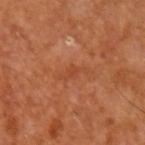workup = total-body-photography surveillance lesion; no biopsy
anatomic site = the left upper arm
lighting = cross-polarized
patient = aged 63 to 67
size = ≈3 mm
imaging modality = 15 mm crop, total-body photography
automated lesion analysis = a color-variation rating of about 0.5/10 and peripheral color asymmetry of about 0